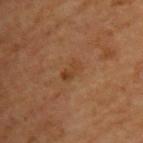image source — ~15 mm tile from a whole-body skin photo | size — about 3 mm | TBP lesion metrics — an average lesion color of about L≈40 a*≈21 b*≈34 (CIELAB), about 6 CIELAB-L* units darker than the surrounding skin, and a lesion-to-skin contrast of about 6 (normalized; higher = more distinct) | body site — the upper back | illumination — cross-polarized | subject — male, aged 63–67.